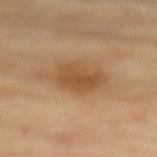Q: Was a biopsy performed?
A: no biopsy performed (imaged during a skin exam)
Q: Who is the patient?
A: female, approximately 80 years of age
Q: What kind of image is this?
A: ~15 mm crop, total-body skin-cancer survey
Q: Lesion location?
A: the mid back